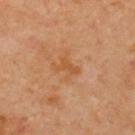follow-up=total-body-photography surveillance lesion; no biopsy
image-analysis metrics=a shape eccentricity near 0.85 and a symmetry-axis asymmetry near 0.5; a normalized lesion–skin contrast near 6; a border-irregularity index near 5.5/10, a within-lesion color-variation index near 0/10, and peripheral color asymmetry of about 0
patient=male, aged around 70
lesion size=about 2.5 mm
location=the upper back
image source=15 mm crop, total-body photography